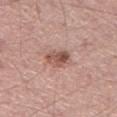follow-up: total-body-photography surveillance lesion; no biopsy
patient: male, approximately 40 years of age
image: ~15 mm tile from a whole-body skin photo
tile lighting: white-light
location: the right lower leg
lesion diameter: ≈3.5 mm